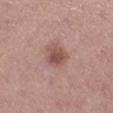Q: Was this lesion biopsied?
A: catalogued during a skin exam; not biopsied
Q: What is the anatomic site?
A: the right lower leg
Q: What did automated image analysis measure?
A: a lesion area of about 6.5 mm², an outline eccentricity of about 0.65 (0 = round, 1 = elongated), and two-axis asymmetry of about 0.2; a lesion color around L≈51 a*≈22 b*≈24 in CIELAB, roughly 11 lightness units darker than nearby skin, and a normalized border contrast of about 7.5
Q: How was this image acquired?
A: total-body-photography crop, ~15 mm field of view
Q: What are the patient's age and sex?
A: female, in their 60s
Q: How large is the lesion?
A: ≈3 mm